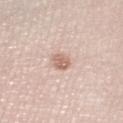<tbp_lesion>
  <automated_metrics>
    <area_mm2_approx>4.0</area_mm2_approx>
    <eccentricity>0.75</eccentricity>
    <cielab_L>65</cielab_L>
    <cielab_a>18</cielab_a>
    <cielab_b>27</cielab_b>
    <vs_skin_darker_L>11.0</vs_skin_darker_L>
    <vs_skin_contrast_norm>7.0</vs_skin_contrast_norm>
  </automated_metrics>
  <lesion_size>
    <long_diameter_mm_approx>2.5</long_diameter_mm_approx>
  </lesion_size>
  <site>right lower leg</site>
  <lighting>white-light</lighting>
  <patient>
    <sex>female</sex>
    <age_approx>60</age_approx>
  </patient>
  <image>
    <source>total-body photography crop</source>
    <field_of_view_mm>15</field_of_view_mm>
  </image>
</tbp_lesion>